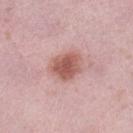notes: no biopsy performed (imaged during a skin exam) | image: 15 mm crop, total-body photography | anatomic site: the left lower leg | tile lighting: white-light | subject: female, aged approximately 40 | size: ~4 mm (longest diameter).